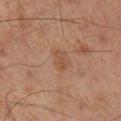{
  "biopsy_status": "not biopsied; imaged during a skin examination",
  "image": {
    "source": "total-body photography crop",
    "field_of_view_mm": 15
  },
  "automated_metrics": {
    "border_irregularity_0_10": 3.0,
    "color_variation_0_10": 1.5,
    "peripheral_color_asymmetry": 0.5
  },
  "site": "leg",
  "patient": {
    "sex": "male",
    "age_approx": 65
  }
}